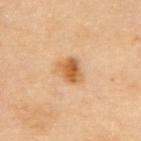The lesion was tiled from a total-body skin photograph and was not biopsied.
Cropped from a whole-body photographic skin survey; the tile spans about 15 mm.
A female subject aged 63 to 67.
On the upper back.
Longest diameter approximately 3 mm.